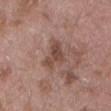The lesion was tiled from a total-body skin photograph and was not biopsied. From the lower back. About 4 mm across. A male subject, aged around 55. A region of skin cropped from a whole-body photographic capture, roughly 15 mm wide. Automated image analysis of the tile measured an area of roughly 7.5 mm² and a symmetry-axis asymmetry near 0.4. The software also gave a border-irregularity rating of about 4.5/10, a color-variation rating of about 3.5/10, and a peripheral color-asymmetry measure near 1. And it measured a classifier nevus-likeness of about 0/100 and lesion-presence confidence of about 100/100.